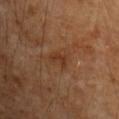Q: Is there a histopathology result?
A: imaged on a skin check; not biopsied